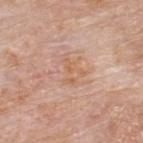workup: total-body-photography surveillance lesion; no biopsy | patient: male, approximately 75 years of age | image source: ~15 mm crop, total-body skin-cancer survey | size: ≈3.5 mm | tile lighting: white-light illumination | location: the back | TBP lesion metrics: a lesion area of about 5 mm², an outline eccentricity of about 0.75 (0 = round, 1 = elongated), and a shape-asymmetry score of about 0.5 (0 = symmetric); a classifier nevus-likeness of about 0/100.